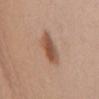Assessment:
This lesion was catalogued during total-body skin photography and was not selected for biopsy.
Context:
A female subject, aged around 40. The lesion-visualizer software estimated a mean CIELAB color near L≈52 a*≈21 b*≈30, a lesion–skin lightness drop of about 12, and a lesion-to-skin contrast of about 8.5 (normalized; higher = more distinct). And it measured a classifier nevus-likeness of about 95/100 and a detector confidence of about 100 out of 100 that the crop contains a lesion. The recorded lesion diameter is about 4.5 mm. Cropped from a total-body skin-imaging series; the visible field is about 15 mm. From the chest.